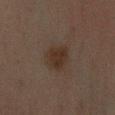Assessment: Recorded during total-body skin imaging; not selected for excision or biopsy. Background: About 3.5 mm across. The lesion is on the right forearm. An algorithmic analysis of the crop reported a footprint of about 8.5 mm², an eccentricity of roughly 0.45, and a symmetry-axis asymmetry near 0.2. A 15 mm crop from a total-body photograph taken for skin-cancer surveillance. The tile uses cross-polarized illumination. A male patient aged around 45.